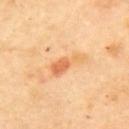Assessment:
Imaged during a routine full-body skin examination; the lesion was not biopsied and no histopathology is available.
Background:
Automated tile analysis of the lesion measured an area of roughly 6 mm², an eccentricity of roughly 0.9, and a shape-asymmetry score of about 0.5 (0 = symmetric). And it measured a mean CIELAB color near L≈70 a*≈26 b*≈45, roughly 11 lightness units darker than nearby skin, and a normalized border contrast of about 6.5. The analysis additionally found a classifier nevus-likeness of about 5/100 and lesion-presence confidence of about 100/100. A region of skin cropped from a whole-body photographic capture, roughly 15 mm wide. The lesion is on the upper back. Captured under cross-polarized illumination. The patient is a female aged approximately 60.